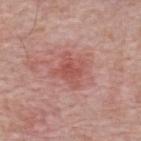| key | value |
|---|---|
| biopsy status | catalogued during a skin exam; not biopsied |
| site | the upper back |
| patient | male, aged approximately 65 |
| imaging modality | 15 mm crop, total-body photography |